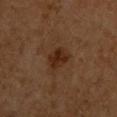Part of a total-body skin-imaging series; this lesion was reviewed on a skin check and was not flagged for biopsy.